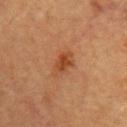Assessment:
Imaged during a routine full-body skin examination; the lesion was not biopsied and no histopathology is available.
Context:
This image is a 15 mm lesion crop taken from a total-body photograph. The lesion is located on the upper back. This is a cross-polarized tile. An algorithmic analysis of the crop reported a footprint of about 6 mm², a shape eccentricity near 0.8, and a symmetry-axis asymmetry near 0.25. The analysis additionally found a mean CIELAB color near L≈38 a*≈22 b*≈32, a lesion–skin lightness drop of about 8, and a normalized lesion–skin contrast near 7. The software also gave a border-irregularity index near 3/10, a color-variation rating of about 3/10, and a peripheral color-asymmetry measure near 1. And it measured a nevus-likeness score of about 75/100 and a detector confidence of about 100 out of 100 that the crop contains a lesion. The subject is a male aged approximately 60. Longest diameter approximately 3.5 mm.